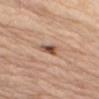Notes:
- workup: imaged on a skin check; not biopsied
- patient: female, in their mid- to late 60s
- body site: the left arm
- image-analysis metrics: an average lesion color of about L≈53 a*≈20 b*≈30 (CIELAB), about 14 CIELAB-L* units darker than the surrounding skin, and a normalized border contrast of about 9
- lesion size: ≈4 mm
- lighting: white-light
- image: total-body-photography crop, ~15 mm field of view